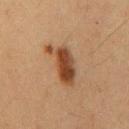follow-up=total-body-photography surveillance lesion; no biopsy | acquisition=~15 mm tile from a whole-body skin photo | location=the front of the torso | subject=male, aged approximately 65.